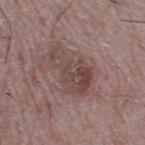This lesion was catalogued during total-body skin photography and was not selected for biopsy.
The tile uses white-light illumination.
Automated tile analysis of the lesion measured a border-irregularity rating of about 6/10, a within-lesion color-variation index near 6.5/10, and a peripheral color-asymmetry measure near 2. The analysis additionally found an automated nevus-likeness rating near 15 out of 100 and a detector confidence of about 100 out of 100 that the crop contains a lesion.
About 6 mm across.
A male patient, aged around 55.
The lesion is on the right thigh.
A 15 mm crop from a total-body photograph taken for skin-cancer surveillance.